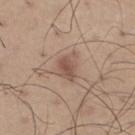| field | value |
|---|---|
| acquisition | 15 mm crop, total-body photography |
| subject | male, aged 63 to 67 |
| tile lighting | white-light illumination |
| body site | the right thigh |